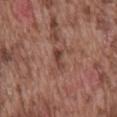No biopsy was performed on this lesion — it was imaged during a full skin examination and was not determined to be concerning. Captured under white-light illumination. Longest diameter approximately 2.5 mm. The patient is a male aged around 75. Located on the mid back. A lesion tile, about 15 mm wide, cut from a 3D total-body photograph.